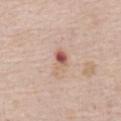Captured under white-light illumination.
The lesion is located on the abdomen.
Cropped from a total-body skin-imaging series; the visible field is about 15 mm.
A female patient, aged around 75.
An algorithmic analysis of the crop reported an eccentricity of roughly 0.85 and a shape-asymmetry score of about 0.45 (0 = symmetric). It also reported a border-irregularity index near 4.5/10, a color-variation rating of about 10/10, and peripheral color asymmetry of about 3.5. The analysis additionally found a nevus-likeness score of about 0/100.
Measured at roughly 3.5 mm in maximum diameter.